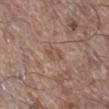This lesion was catalogued during total-body skin photography and was not selected for biopsy. A region of skin cropped from a whole-body photographic capture, roughly 15 mm wide. Located on the right lower leg. The patient is a male aged approximately 65.